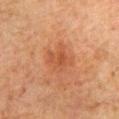Assessment:
The lesion was photographed on a routine skin check and not biopsied; there is no pathology result.
Background:
A male patient, aged 78 to 82. Imaged with cross-polarized lighting. A close-up tile cropped from a whole-body skin photograph, about 15 mm across. The lesion is on the abdomen.